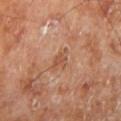Assessment: Recorded during total-body skin imaging; not selected for excision or biopsy. Background: This is a cross-polarized tile. A roughly 15 mm field-of-view crop from a total-body skin photograph. The lesion's longest dimension is about 2.5 mm. Automated tile analysis of the lesion measured an average lesion color of about L≈50 a*≈22 b*≈32 (CIELAB) and a lesion–skin lightness drop of about 8. And it measured a border-irregularity rating of about 3/10 and a within-lesion color-variation index near 1/10. The analysis additionally found lesion-presence confidence of about 100/100. The lesion is on the left lower leg. A male subject, aged 68 to 72.